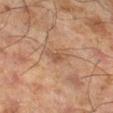  biopsy_status: not biopsied; imaged during a skin examination
  automated_metrics:
    area_mm2_approx: 3.5
    eccentricity: 0.75
    border_irregularity_0_10: 4.5
    color_variation_0_10: 2.0
    peripheral_color_asymmetry: 0.5
  image:
    source: total-body photography crop
    field_of_view_mm: 15
  lesion_size:
    long_diameter_mm_approx: 3.0
  lighting: cross-polarized
  patient:
    sex: male
    age_approx: 65
  site: left lower leg